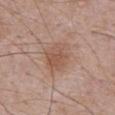follow-up: catalogued during a skin exam; not biopsied
automated lesion analysis: a footprint of about 11 mm², an outline eccentricity of about 0.6 (0 = round, 1 = elongated), and a symmetry-axis asymmetry near 0.2; an average lesion color of about L≈54 a*≈20 b*≈28 (CIELAB), a lesion–skin lightness drop of about 8, and a normalized lesion–skin contrast near 6; internal color variation of about 3.5 on a 0–10 scale and radial color variation of about 1; an automated nevus-likeness rating near 65 out of 100
site: the abdomen
diameter: about 4 mm
image: 15 mm crop, total-body photography
patient: male, approximately 70 years of age